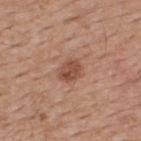Findings:
* workup · total-body-photography surveillance lesion; no biopsy
* subject · male, approximately 50 years of age
* tile lighting · white-light
* image-analysis metrics · an average lesion color of about L≈49 a*≈23 b*≈29 (CIELAB) and a normalized lesion–skin contrast near 7.5
* diameter · about 3 mm
* location · the upper back
* image source · ~15 mm tile from a whole-body skin photo